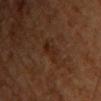Part of a total-body skin-imaging series; this lesion was reviewed on a skin check and was not flagged for biopsy.
On the back.
Automated image analysis of the tile measured an outline eccentricity of about 0.85 (0 = round, 1 = elongated).
This is a cross-polarized tile.
A lesion tile, about 15 mm wide, cut from a 3D total-body photograph.
A male patient roughly 60 years of age.
Approximately 3.5 mm at its widest.